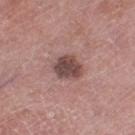biopsy status: catalogued during a skin exam; not biopsied | patient: female, about 70 years old | lighting: white-light | TBP lesion metrics: an area of roughly 7.5 mm², an eccentricity of roughly 0.6, and two-axis asymmetry of about 0.2; about 14 CIELAB-L* units darker than the surrounding skin and a normalized lesion–skin contrast near 10; border irregularity of about 2 on a 0–10 scale and peripheral color asymmetry of about 1 | image source: 15 mm crop, total-body photography | location: the left thigh.